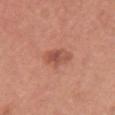Q: Was this lesion biopsied?
A: imaged on a skin check; not biopsied
Q: What lighting was used for the tile?
A: white-light illumination
Q: What are the patient's age and sex?
A: female, aged 33 to 37
Q: Lesion size?
A: ~3.5 mm (longest diameter)
Q: What is the imaging modality?
A: 15 mm crop, total-body photography
Q: Lesion location?
A: the back
Q: Automated lesion metrics?
A: an area of roughly 5.5 mm², an eccentricity of roughly 0.8, and a shape-asymmetry score of about 0.2 (0 = symmetric); a mean CIELAB color near L≈52 a*≈26 b*≈30, a lesion–skin lightness drop of about 9, and a normalized lesion–skin contrast near 6.5; a border-irregularity rating of about 2/10